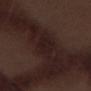The lesion was photographed on a routine skin check and not biopsied; there is no pathology result.
A male subject, aged 68 to 72.
This is a white-light tile.
This image is a 15 mm lesion crop taken from a total-body photograph.
The lesion is on the left thigh.
About 2.5 mm across.